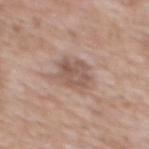workup=imaged on a skin check; not biopsied
image=~15 mm crop, total-body skin-cancer survey
diameter=≈3.5 mm
subject=male, aged approximately 60
site=the front of the torso
TBP lesion metrics=a color-variation rating of about 4/10 and a peripheral color-asymmetry measure near 1.5
illumination=white-light